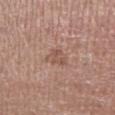biopsy_status: not biopsied; imaged during a skin examination
site: left lower leg
lighting: white-light
automated_metrics:
  cielab_L: 53
  cielab_a: 20
  cielab_b: 26
  vs_skin_contrast_norm: 5.5
  border_irregularity_0_10: 2.5
  color_variation_0_10: 2.0
  peripheral_color_asymmetry: 0.5
lesion_size:
  long_diameter_mm_approx: 2.5
image:
  source: total-body photography crop
  field_of_view_mm: 15
patient:
  sex: male
  age_approx: 70